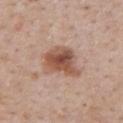  site: upper back
  patient:
    sex: male
    age_approx: 75
  lesion_size:
    long_diameter_mm_approx: 5.0
  image:
    source: total-body photography crop
    field_of_view_mm: 15
  automated_metrics:
    border_irregularity_0_10: 3.5
    color_variation_0_10: 6.5
    peripheral_color_asymmetry: 2.0